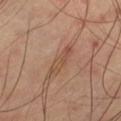| feature | finding |
|---|---|
| biopsy status | no biopsy performed (imaged during a skin exam) |
| subject | male, in their mid- to late 60s |
| automated metrics | a shape eccentricity near 0.95 and two-axis asymmetry of about 0.3; an average lesion color of about L≈49 a*≈20 b*≈30 (CIELAB), about 7 CIELAB-L* units darker than the surrounding skin, and a normalized lesion–skin contrast near 5.5; a border-irregularity index near 3.5/10, a within-lesion color-variation index near 3/10, and a peripheral color-asymmetry measure near 1 |
| acquisition | 15 mm crop, total-body photography |
| lighting | cross-polarized |
| location | the left thigh |